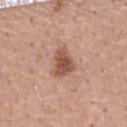Impression:
The lesion was photographed on a routine skin check and not biopsied; there is no pathology result.
Clinical summary:
Cropped from a whole-body photographic skin survey; the tile spans about 15 mm. Automated image analysis of the tile measured a footprint of about 7 mm², a shape eccentricity near 0.65, and a symmetry-axis asymmetry near 0.35. It also reported a lesion-detection confidence of about 100/100. Imaged with white-light lighting. About 3.5 mm across. On the chest. The subject is a male aged around 55.